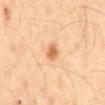- workup · no biopsy performed (imaged during a skin exam)
- illumination · cross-polarized
- subject · male, about 40 years old
- lesion diameter · ~2.5 mm (longest diameter)
- anatomic site · the mid back
- automated metrics · an area of roughly 3.5 mm², an eccentricity of roughly 0.75, and two-axis asymmetry of about 0.3; an average lesion color of about L≈68 a*≈24 b*≈42 (CIELAB) and a normalized border contrast of about 8; a color-variation rating of about 2.5/10 and peripheral color asymmetry of about 1; a lesion-detection confidence of about 100/100
- acquisition · ~15 mm tile from a whole-body skin photo